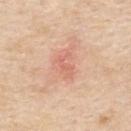Clinical summary:
Longest diameter approximately 3 mm. The lesion is located on the upper back. A 15 mm close-up extracted from a 3D total-body photography capture. The total-body-photography lesion software estimated a footprint of about 4 mm², an eccentricity of roughly 0.8, and two-axis asymmetry of about 0.45. The analysis additionally found a border-irregularity rating of about 6/10. And it measured a nevus-likeness score of about 5/100 and lesion-presence confidence of about 100/100. The subject is a female aged 68–72. This is a white-light tile.